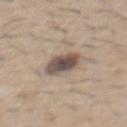Q: Was this lesion biopsied?
A: imaged on a skin check; not biopsied
Q: What are the patient's age and sex?
A: male, aged around 60
Q: Lesion location?
A: the front of the torso
Q: How was this image acquired?
A: 15 mm crop, total-body photography
Q: What lighting was used for the tile?
A: white-light illumination
Q: Automated lesion metrics?
A: an area of roughly 8 mm², an outline eccentricity of about 0.5 (0 = round, 1 = elongated), and a symmetry-axis asymmetry near 0.15; a nevus-likeness score of about 40/100